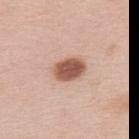Notes:
* workup · total-body-photography surveillance lesion; no biopsy
* lesion size · ≈3.5 mm
* TBP lesion metrics · a lesion area of about 8 mm², an eccentricity of roughly 0.7, and a symmetry-axis asymmetry near 0.1; a mean CIELAB color near L≈55 a*≈22 b*≈29, a lesion–skin lightness drop of about 17, and a normalized lesion–skin contrast near 10.5; a classifier nevus-likeness of about 100/100 and lesion-presence confidence of about 100/100
* illumination · white-light illumination
* location · the upper back
* subject · female, aged 63–67
* image source · total-body-photography crop, ~15 mm field of view